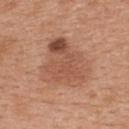Recorded during total-body skin imaging; not selected for excision or biopsy. Captured under white-light illumination. Located on the upper back. About 7 mm across. A close-up tile cropped from a whole-body skin photograph, about 15 mm across. The patient is a male aged approximately 30.